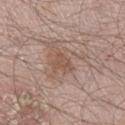Q: Is there a histopathology result?
A: no biopsy performed (imaged during a skin exam)
Q: Where on the body is the lesion?
A: the right lower leg
Q: What is the imaging modality?
A: 15 mm crop, total-body photography
Q: Lesion size?
A: about 2.5 mm
Q: What are the patient's age and sex?
A: male, approximately 60 years of age
Q: Automated lesion metrics?
A: an area of roughly 3.5 mm²; a color-variation rating of about 1/10 and peripheral color asymmetry of about 0.5